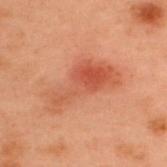Clinical impression: This lesion was catalogued during total-body skin photography and was not selected for biopsy. Background: Located on the upper back. The patient is a male aged around 55. A lesion tile, about 15 mm wide, cut from a 3D total-body photograph.